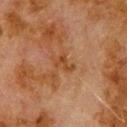Part of a total-body skin-imaging series; this lesion was reviewed on a skin check and was not flagged for biopsy. The lesion is on the upper back. Cropped from a total-body skin-imaging series; the visible field is about 15 mm. Captured under cross-polarized illumination. Automated image analysis of the tile measured an automated nevus-likeness rating near 0 out of 100 and a detector confidence of about 100 out of 100 that the crop contains a lesion. The recorded lesion diameter is about 2.5 mm. A male patient, aged approximately 80.